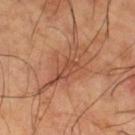Part of a total-body skin-imaging series; this lesion was reviewed on a skin check and was not flagged for biopsy.
A male patient, aged approximately 65.
The lesion is located on the right thigh.
A close-up tile cropped from a whole-body skin photograph, about 15 mm across.